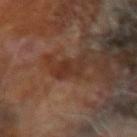Notes:
• notes: total-body-photography surveillance lesion; no biopsy
• subject: male, aged 63 to 67
• imaging modality: ~15 mm tile from a whole-body skin photo
• location: the right forearm